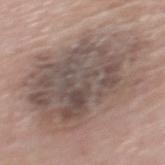Image and clinical context: A male subject aged 63–67. A roughly 15 mm field-of-view crop from a total-body skin photograph. The lesion is on the mid back.